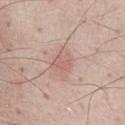{"biopsy_status": "not biopsied; imaged during a skin examination", "site": "front of the torso", "patient": {"sex": "male", "age_approx": 60}, "image": {"source": "total-body photography crop", "field_of_view_mm": 15}}